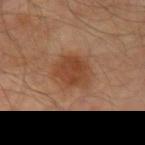follow-up: imaged on a skin check; not biopsied
subject: male, in their mid-60s
TBP lesion metrics: a footprint of about 14 mm² and a symmetry-axis asymmetry near 0.2; a border-irregularity index near 2/10 and a within-lesion color-variation index near 3.5/10; lesion-presence confidence of about 100/100
location: the left upper arm
image: ~15 mm tile from a whole-body skin photo
lesion size: ~4.5 mm (longest diameter)
illumination: cross-polarized illumination